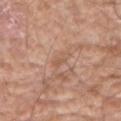- biopsy status — no biopsy performed (imaged during a skin exam)
- patient — male, in their 60s
- image-analysis metrics — a lesion area of about 2.5 mm², a shape eccentricity near 0.95, and a shape-asymmetry score of about 0.4 (0 = symmetric); an automated nevus-likeness rating near 0 out of 100 and a lesion-detection confidence of about 65/100
- acquisition — ~15 mm crop, total-body skin-cancer survey
- body site — the left upper arm
- lesion size — ~3 mm (longest diameter)